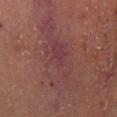Q: Was this lesion biopsied?
A: catalogued during a skin exam; not biopsied
Q: Automated lesion metrics?
A: a footprint of about 27 mm², a shape eccentricity near 0.9, and a shape-asymmetry score of about 0.5 (0 = symmetric); a lesion color around L≈34 a*≈20 b*≈17 in CIELAB and roughly 4 lightness units darker than nearby skin
Q: Patient demographics?
A: male, aged 58 to 62
Q: Illumination type?
A: cross-polarized
Q: What is the lesion's diameter?
A: ~10 mm (longest diameter)
Q: How was this image acquired?
A: ~15 mm tile from a whole-body skin photo